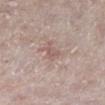| feature | finding |
|---|---|
| workup | catalogued during a skin exam; not biopsied |
| size | ~2.5 mm (longest diameter) |
| illumination | white-light |
| imaging modality | ~15 mm crop, total-body skin-cancer survey |
| subject | female, aged 68 to 72 |
| anatomic site | the left lower leg |